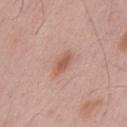Approximately 3 mm at its widest. The lesion-visualizer software estimated a lesion area of about 4 mm² and a shape-asymmetry score of about 0.3 (0 = symmetric). The software also gave an average lesion color of about L≈57 a*≈23 b*≈28 (CIELAB). And it measured a classifier nevus-likeness of about 70/100 and a lesion-detection confidence of about 100/100. The patient is a male aged 53 to 57. A 15 mm close-up extracted from a 3D total-body photography capture. From the mid back.